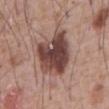workup — total-body-photography surveillance lesion; no biopsy | size — about 6 mm | site — the chest | lighting — white-light | patient — male, in their 60s | acquisition — 15 mm crop, total-body photography.